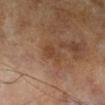Q: Is there a histopathology result?
A: total-body-photography surveillance lesion; no biopsy
Q: Who is the patient?
A: male, about 65 years old
Q: How large is the lesion?
A: ≈3 mm
Q: What lighting was used for the tile?
A: cross-polarized illumination
Q: What kind of image is this?
A: total-body-photography crop, ~15 mm field of view